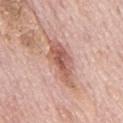follow-up=imaged on a skin check; not biopsied | patient=male, aged around 75 | imaging modality=total-body-photography crop, ~15 mm field of view | body site=the mid back.